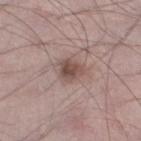workup: catalogued during a skin exam; not biopsied | acquisition: 15 mm crop, total-body photography | anatomic site: the left lower leg | subject: male, aged approximately 70 | tile lighting: white-light | automated lesion analysis: a footprint of about 5.5 mm², an eccentricity of roughly 0.45, and a shape-asymmetry score of about 0.3 (0 = symmetric); a mean CIELAB color near L≈49 a*≈17 b*≈21, about 11 CIELAB-L* units darker than the surrounding skin, and a lesion-to-skin contrast of about 8.5 (normalized; higher = more distinct); a border-irregularity index near 2.5/10, internal color variation of about 4 on a 0–10 scale, and a peripheral color-asymmetry measure near 1.5; a nevus-likeness score of about 75/100 and a lesion-detection confidence of about 100/100.